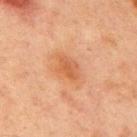<case>
<image>
  <source>total-body photography crop</source>
  <field_of_view_mm>15</field_of_view_mm>
</image>
<lesion_size>
  <long_diameter_mm_approx>3.5</long_diameter_mm_approx>
</lesion_size>
<automated_metrics>
  <cielab_L>47</cielab_L>
  <cielab_a>22</cielab_a>
  <cielab_b>33</cielab_b>
  <color_variation_0_10>2.0</color_variation_0_10>
  <peripheral_color_asymmetry>0.5</peripheral_color_asymmetry>
</automated_metrics>
<patient>
  <sex>male</sex>
  <age_approx>65</age_approx>
</patient>
<site>chest</site>
<lighting>cross-polarized</lighting>
</case>